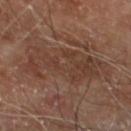Notes:
- follow-up: catalogued during a skin exam; not biopsied
- image: ~15 mm tile from a whole-body skin photo
- patient: male, aged around 70
- lighting: cross-polarized illumination
- site: the left lower leg
- diameter: ~9.5 mm (longest diameter)